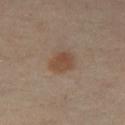{
  "biopsy_status": "not biopsied; imaged during a skin examination",
  "lighting": "cross-polarized",
  "image": {
    "source": "total-body photography crop",
    "field_of_view_mm": 15
  },
  "automated_metrics": {
    "cielab_L": 47,
    "cielab_a": 17,
    "cielab_b": 28,
    "vs_skin_darker_L": 8.0,
    "vs_skin_contrast_norm": 7.5
  },
  "site": "left leg",
  "patient": {
    "sex": "female",
    "age_approx": 40
  },
  "lesion_size": {
    "long_diameter_mm_approx": 3.5
  }
}